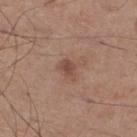Case summary:
– workup · total-body-photography surveillance lesion; no biopsy
– body site · the right lower leg
– lesion diameter · ~2.5 mm (longest diameter)
– illumination · white-light
– imaging modality · total-body-photography crop, ~15 mm field of view
– subject · male, approximately 60 years of age
– automated lesion analysis · a lesion area of about 3.5 mm², a shape eccentricity near 0.7, and two-axis asymmetry of about 0.3; border irregularity of about 3 on a 0–10 scale, internal color variation of about 2 on a 0–10 scale, and radial color variation of about 0.5; a nevus-likeness score of about 5/100 and a lesion-detection confidence of about 100/100